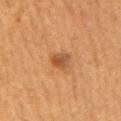biopsy status=imaged on a skin check; not biopsied
image source=15 mm crop, total-body photography
subject=male, about 55 years old
site=the right upper arm